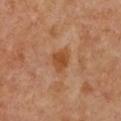biopsy_status: not biopsied; imaged during a skin examination